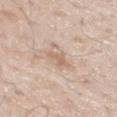| key | value |
|---|---|
| biopsy status | total-body-photography surveillance lesion; no biopsy |
| automated metrics | an area of roughly 3.5 mm², a shape eccentricity near 0.7, and two-axis asymmetry of about 0.4; a mean CIELAB color near L≈64 a*≈16 b*≈29, a lesion–skin lightness drop of about 8, and a normalized border contrast of about 5.5; a border-irregularity rating of about 4/10, internal color variation of about 0.5 on a 0–10 scale, and radial color variation of about 0.5; a classifier nevus-likeness of about 0/100 and a lesion-detection confidence of about 100/100 |
| patient | male, approximately 55 years of age |
| acquisition | total-body-photography crop, ~15 mm field of view |
| size | about 2.5 mm |
| body site | the right upper arm |
| illumination | white-light |